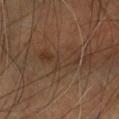| feature | finding |
|---|---|
| notes | catalogued during a skin exam; not biopsied |
| location | the arm |
| imaging modality | ~15 mm crop, total-body skin-cancer survey |
| lighting | cross-polarized illumination |
| lesion size | about 5.5 mm |
| patient | male, aged approximately 60 |
| automated metrics | a lesion–skin lightness drop of about 5 and a lesion-to-skin contrast of about 4.5 (normalized; higher = more distinct) |